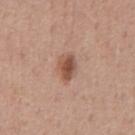| feature | finding |
|---|---|
| anatomic site | the abdomen |
| automated lesion analysis | a border-irregularity index near 2/10, a color-variation rating of about 5/10, and a peripheral color-asymmetry measure near 1.5; a classifier nevus-likeness of about 90/100 and lesion-presence confidence of about 100/100 |
| patient | male, aged around 50 |
| diameter | about 3 mm |
| imaging modality | total-body-photography crop, ~15 mm field of view |
| lighting | white-light |